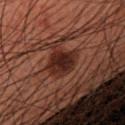follow-up — catalogued during a skin exam; not biopsied
site — the right forearm
subject — male, aged 58–62
acquisition — 15 mm crop, total-body photography